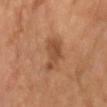{"patient": {"sex": "male", "age_approx": 65}, "image": {"source": "total-body photography crop", "field_of_view_mm": 15}}